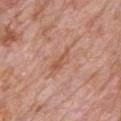This lesion was catalogued during total-body skin photography and was not selected for biopsy. The lesion is on the chest. A lesion tile, about 15 mm wide, cut from a 3D total-body photograph. The subject is a male aged 78–82.